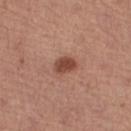Notes:
* follow-up: catalogued during a skin exam; not biopsied
* image-analysis metrics: about 12 CIELAB-L* units darker than the surrounding skin and a normalized lesion–skin contrast near 8.5; a border-irregularity rating of about 2/10, a color-variation rating of about 2/10, and a peripheral color-asymmetry measure near 1
* imaging modality: ~15 mm tile from a whole-body skin photo
* subject: female, in their mid-60s
* tile lighting: white-light illumination
* anatomic site: the right thigh